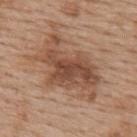Q: Is there a histopathology result?
A: imaged on a skin check; not biopsied
Q: Where on the body is the lesion?
A: the upper back
Q: What is the lesion's diameter?
A: ≈9 mm
Q: What lighting was used for the tile?
A: white-light illumination
Q: Automated lesion metrics?
A: border irregularity of about 6 on a 0–10 scale and a within-lesion color-variation index near 6/10; an automated nevus-likeness rating near 45 out of 100 and a detector confidence of about 100 out of 100 that the crop contains a lesion
Q: What is the imaging modality?
A: ~15 mm crop, total-body skin-cancer survey
Q: What are the patient's age and sex?
A: female, aged 63–67